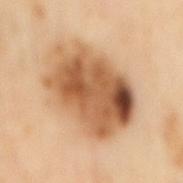Measured at roughly 8.5 mm in maximum diameter.
The total-body-photography lesion software estimated a border-irregularity rating of about 2/10, a within-lesion color-variation index near 10/10, and a peripheral color-asymmetry measure near 4.5.
A male patient roughly 65 years of age.
A region of skin cropped from a whole-body photographic capture, roughly 15 mm wide.
Captured under cross-polarized illumination.
On the mid back.